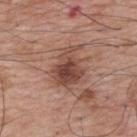Captured during whole-body skin photography for melanoma surveillance; the lesion was not biopsied. Located on the upper back. Captured under white-light illumination. Cropped from a whole-body photographic skin survey; the tile spans about 15 mm. A male patient aged 68–72.